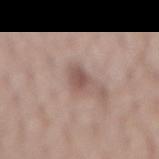{"biopsy_status": "not biopsied; imaged during a skin examination", "site": "mid back", "patient": {"sex": "male", "age_approx": 60}, "lesion_size": {"long_diameter_mm_approx": 3.0}, "image": {"source": "total-body photography crop", "field_of_view_mm": 15}, "automated_metrics": {"area_mm2_approx": 5.0, "eccentricity": 0.7, "shape_asymmetry": 0.35, "cielab_L": 53, "cielab_a": 17, "cielab_b": 22, "vs_skin_darker_L": 10.0}}